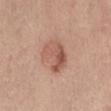Image and clinical context:
Automated tile analysis of the lesion measured an area of roughly 12 mm², a shape eccentricity near 0.7, and two-axis asymmetry of about 0.15. A female subject aged 53–57. A close-up tile cropped from a whole-body skin photograph, about 15 mm across. The lesion is on the abdomen. Longest diameter approximately 4.5 mm. The tile uses white-light illumination.